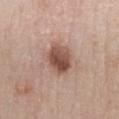<lesion>
  <biopsy_status>not biopsied; imaged during a skin examination</biopsy_status>
  <patient>
    <sex>female</sex>
    <age_approx>45</age_approx>
  </patient>
  <site>chest</site>
  <automated_metrics>
    <area_mm2_approx>10.0</area_mm2_approx>
    <eccentricity>0.6</eccentricity>
    <shape_asymmetry>0.1</shape_asymmetry>
    <cielab_L>51</cielab_L>
    <cielab_a>21</cielab_a>
    <cielab_b>27</cielab_b>
    <vs_skin_darker_L>14.0</vs_skin_darker_L>
    <vs_skin_contrast_norm>9.5</vs_skin_contrast_norm>
    <peripheral_color_asymmetry>2.5</peripheral_color_asymmetry>
    <lesion_detection_confidence_0_100>100</lesion_detection_confidence_0_100>
  </automated_metrics>
  <lesion_size>
    <long_diameter_mm_approx>4.0</long_diameter_mm_approx>
  </lesion_size>
  <image>
    <source>total-body photography crop</source>
    <field_of_view_mm>15</field_of_view_mm>
  </image>
  <lighting>white-light</lighting>
</lesion>